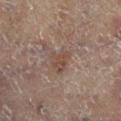Clinical impression:
The lesion was photographed on a routine skin check and not biopsied; there is no pathology result.
Acquisition and patient details:
The lesion is located on the right leg. Cropped from a whole-body photographic skin survey; the tile spans about 15 mm. The tile uses cross-polarized illumination. The recorded lesion diameter is about 3 mm. A female subject, about 80 years old.